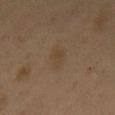biopsy status: total-body-photography surveillance lesion; no biopsy
anatomic site: the chest
lesion size: about 3.5 mm
subject: male, aged 48 to 52
image source: total-body-photography crop, ~15 mm field of view
lighting: cross-polarized illumination